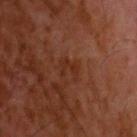biopsy status: imaged on a skin check; not biopsied
TBP lesion metrics: a lesion color around L≈28 a*≈22 b*≈28 in CIELAB, a lesion–skin lightness drop of about 5, and a normalized lesion–skin contrast near 5; an automated nevus-likeness rating near 0 out of 100 and a detector confidence of about 100 out of 100 that the crop contains a lesion
lighting: cross-polarized illumination
lesion diameter: ~3 mm (longest diameter)
subject: male, aged around 60
imaging modality: ~15 mm tile from a whole-body skin photo
body site: the head or neck